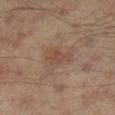| feature | finding |
|---|---|
| body site | the leg |
| subject | male, roughly 65 years of age |
| automated metrics | an average lesion color of about L≈44 a*≈18 b*≈27 (CIELAB) |
| imaging modality | ~15 mm crop, total-body skin-cancer survey |
| lesion diameter | ≈3 mm |
| lighting | cross-polarized illumination |